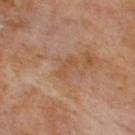<case>
<biopsy_status>not biopsied; imaged during a skin examination</biopsy_status>
<patient>
  <sex>male</sex>
  <age_approx>70</age_approx>
</patient>
<site>upper back</site>
<automated_metrics>
  <cielab_L>50</cielab_L>
  <cielab_a>20</cielab_a>
  <cielab_b>33</cielab_b>
  <vs_skin_darker_L>5.0</vs_skin_darker_L>
  <vs_skin_contrast_norm>5.0</vs_skin_contrast_norm>
  <border_irregularity_0_10>3.5</border_irregularity_0_10>
  <color_variation_0_10>0.5</color_variation_0_10>
</automated_metrics>
<lighting>cross-polarized</lighting>
<image>
  <source>total-body photography crop</source>
  <field_of_view_mm>15</field_of_view_mm>
</image>
</case>